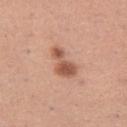biopsy_status: not biopsied; imaged during a skin examination
lesion_size:
  long_diameter_mm_approx: 4.0
automated_metrics:
  cielab_L: 56
  cielab_a: 24
  cielab_b: 31
  vs_skin_darker_L: 13.0
  vs_skin_contrast_norm: 8.5
patient:
  sex: female
  age_approx: 30
image:
  source: total-body photography crop
  field_of_view_mm: 15
lighting: white-light
site: left thigh